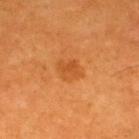Q: Is there a histopathology result?
A: no biopsy performed (imaged during a skin exam)
Q: Lesion location?
A: the back
Q: What is the lesion's diameter?
A: about 3 mm
Q: What is the imaging modality?
A: total-body-photography crop, ~15 mm field of view
Q: What are the patient's age and sex?
A: male, aged 58–62
Q: How was the tile lit?
A: cross-polarized illumination